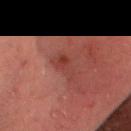Part of a total-body skin-imaging series; this lesion was reviewed on a skin check and was not flagged for biopsy.
The subject is a male aged 58 to 62.
Located on the head or neck.
The lesion-visualizer software estimated two-axis asymmetry of about 0.4. The software also gave an average lesion color of about L≈30 a*≈22 b*≈21 (CIELAB), about 6 CIELAB-L* units darker than the surrounding skin, and a normalized lesion–skin contrast near 5.5. The software also gave border irregularity of about 4.5 on a 0–10 scale, internal color variation of about 3.5 on a 0–10 scale, and radial color variation of about 1.
A close-up tile cropped from a whole-body skin photograph, about 15 mm across.